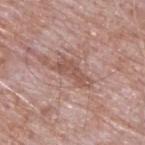Recorded during total-body skin imaging; not selected for excision or biopsy. This is a white-light tile. A male subject aged around 65. From the upper back. The lesion's longest dimension is about 4.5 mm. An algorithmic analysis of the crop reported a border-irregularity index near 5/10, a within-lesion color-variation index near 2/10, and radial color variation of about 0.5. It also reported an automated nevus-likeness rating near 0 out of 100 and a detector confidence of about 100 out of 100 that the crop contains a lesion. A roughly 15 mm field-of-view crop from a total-body skin photograph.